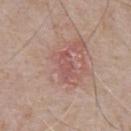Impression:
Captured during whole-body skin photography for melanoma surveillance; the lesion was not biopsied.
Image and clinical context:
The lesion's longest dimension is about 3 mm. On the chest. A 15 mm close-up tile from a total-body photography series done for melanoma screening. A male subject, aged around 80.